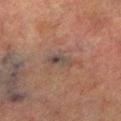biopsy status=catalogued during a skin exam; not biopsied
location=the left lower leg
image source=15 mm crop, total-body photography
patient=male, in their mid- to late 70s
image-analysis metrics=an eccentricity of roughly 0.9 and a symmetry-axis asymmetry near 0.25; an average lesion color of about L≈37 a*≈13 b*≈19 (CIELAB), a lesion–skin lightness drop of about 6, and a normalized lesion–skin contrast near 6.5
size=about 4 mm
lighting=cross-polarized illumination